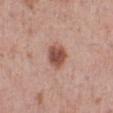Q: Is there a histopathology result?
A: catalogued during a skin exam; not biopsied
Q: Patient demographics?
A: female, approximately 40 years of age
Q: What is the imaging modality?
A: ~15 mm tile from a whole-body skin photo
Q: Lesion location?
A: the left lower leg